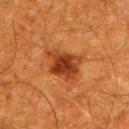Q: Was a biopsy performed?
A: no biopsy performed (imaged during a skin exam)
Q: Patient demographics?
A: male, aged around 60
Q: Lesion location?
A: the upper back
Q: How was the tile lit?
A: cross-polarized
Q: Lesion size?
A: about 4.5 mm
Q: How was this image acquired?
A: ~15 mm crop, total-body skin-cancer survey